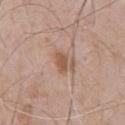The lesion was photographed on a routine skin check and not biopsied; there is no pathology result. A 15 mm crop from a total-body photograph taken for skin-cancer surveillance. Longest diameter approximately 2.5 mm. From the chest. This is a white-light tile. A male patient roughly 50 years of age.